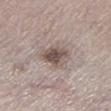Q: Was a biopsy performed?
A: catalogued during a skin exam; not biopsied
Q: What did automated image analysis measure?
A: a lesion color around L≈51 a*≈13 b*≈20 in CIELAB, a lesion–skin lightness drop of about 12, and a normalized border contrast of about 9; a border-irregularity index near 3/10 and radial color variation of about 1.5; a nevus-likeness score of about 40/100 and a detector confidence of about 100 out of 100 that the crop contains a lesion
Q: What are the patient's age and sex?
A: male, roughly 80 years of age
Q: Where on the body is the lesion?
A: the left lower leg
Q: What is the lesion's diameter?
A: about 3.5 mm
Q: How was this image acquired?
A: total-body-photography crop, ~15 mm field of view